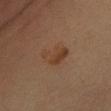Clinical impression: This lesion was catalogued during total-body skin photography and was not selected for biopsy. Context: A male patient in their 60s. From the mid back. This image is a 15 mm lesion crop taken from a total-body photograph. Imaged with cross-polarized lighting. About 4 mm across. The lesion-visualizer software estimated an outline eccentricity of about 0.85 (0 = round, 1 = elongated) and two-axis asymmetry of about 0.4. The software also gave a border-irregularity rating of about 4.5/10, a color-variation rating of about 3.5/10, and radial color variation of about 1.5. The analysis additionally found an automated nevus-likeness rating near 55 out of 100.